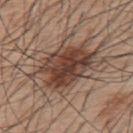• follow-up — catalogued during a skin exam; not biopsied
• anatomic site — the upper back
• image source — ~15 mm crop, total-body skin-cancer survey
• subject — male, aged 53 to 57